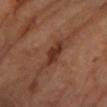Recorded during total-body skin imaging; not selected for excision or biopsy. A roughly 15 mm field-of-view crop from a total-body skin photograph. About 5 mm across. A female subject aged around 60. Automated tile analysis of the lesion measured a footprint of about 7 mm² and two-axis asymmetry of about 0.3. It also reported an average lesion color of about L≈36 a*≈23 b*≈29 (CIELAB), a lesion–skin lightness drop of about 10, and a normalized border contrast of about 8.5. The software also gave a border-irregularity rating of about 4/10, internal color variation of about 2.5 on a 0–10 scale, and a peripheral color-asymmetry measure near 0.5. It also reported a classifier nevus-likeness of about 5/100. On the left forearm.